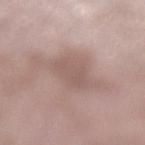Impression: Imaged during a routine full-body skin examination; the lesion was not biopsied and no histopathology is available. Clinical summary: A female subject, approximately 70 years of age. About 4.5 mm across. An algorithmic analysis of the crop reported an area of roughly 8 mm², an outline eccentricity of about 0.85 (0 = round, 1 = elongated), and a shape-asymmetry score of about 0.25 (0 = symmetric). It also reported a lesion color around L≈56 a*≈17 b*≈22 in CIELAB, about 7 CIELAB-L* units darker than the surrounding skin, and a normalized border contrast of about 5. It also reported a classifier nevus-likeness of about 0/100 and lesion-presence confidence of about 60/100. The tile uses white-light illumination. On the left lower leg. A 15 mm crop from a total-body photograph taken for skin-cancer surveillance.